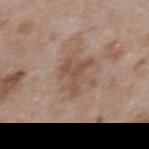Captured during whole-body skin photography for melanoma surveillance; the lesion was not biopsied. The total-body-photography lesion software estimated an average lesion color of about L≈51 a*≈17 b*≈27 (CIELAB) and a lesion-to-skin contrast of about 6 (normalized; higher = more distinct). And it measured a border-irregularity index near 8/10, a color-variation rating of about 1.5/10, and a peripheral color-asymmetry measure near 0.5. And it measured an automated nevus-likeness rating near 0 out of 100 and a lesion-detection confidence of about 100/100. The patient is a female aged 28 to 32. The recorded lesion diameter is about 4 mm. Captured under white-light illumination. Located on the upper back. Cropped from a total-body skin-imaging series; the visible field is about 15 mm.